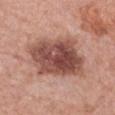Imaged during a routine full-body skin examination; the lesion was not biopsied and no histopathology is available. The subject is a female aged around 60. Approximately 7.5 mm at its widest. On the chest. A 15 mm close-up extracted from a 3D total-body photography capture.